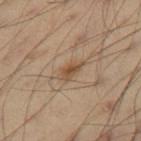<record>
<biopsy_status>not biopsied; imaged during a skin examination</biopsy_status>
<lighting>cross-polarized</lighting>
<patient>
  <sex>male</sex>
  <age_approx>40</age_approx>
</patient>
<lesion_size>
  <long_diameter_mm_approx>3.0</long_diameter_mm_approx>
</lesion_size>
<site>right thigh</site>
<image>
  <source>total-body photography crop</source>
  <field_of_view_mm>15</field_of_view_mm>
</image>
<automated_metrics>
  <area_mm2_approx>4.0</area_mm2_approx>
  <eccentricity>0.85</eccentricity>
  <shape_asymmetry>0.3</shape_asymmetry>
  <cielab_L>50</cielab_L>
  <cielab_a>16</cielab_a>
  <cielab_b>31</cielab_b>
  <vs_skin_darker_L>9.0</vs_skin_darker_L>
  <vs_skin_contrast_norm>7.0</vs_skin_contrast_norm>
</automated_metrics>
</record>